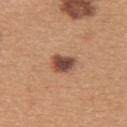notes — catalogued during a skin exam; not biopsied
subject — female, approximately 30 years of age
site — the back
lesion diameter — about 3 mm
imaging modality — 15 mm crop, total-body photography
tile lighting — white-light illumination